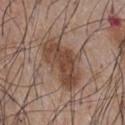Assessment: This lesion was catalogued during total-body skin photography and was not selected for biopsy. Context: A lesion tile, about 15 mm wide, cut from a 3D total-body photograph. The lesion's longest dimension is about 6.5 mm. A male subject aged 53 to 57. Automated tile analysis of the lesion measured a border-irregularity rating of about 4/10 and radial color variation of about 1.5. Captured under white-light illumination. On the chest.